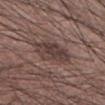The lesion is on the right forearm. Imaged with white-light lighting. The patient is a male in their mid-50s. The recorded lesion diameter is about 4.5 mm. Cropped from a total-body skin-imaging series; the visible field is about 15 mm.